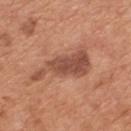Part of a total-body skin-imaging series; this lesion was reviewed on a skin check and was not flagged for biopsy.
Imaged with white-light lighting.
A male subject, aged approximately 65.
Approximately 8 mm at its widest.
A 15 mm crop from a total-body photograph taken for skin-cancer surveillance.
The lesion is located on the mid back.
An algorithmic analysis of the crop reported an area of roughly 15 mm², a shape eccentricity near 0.95, and two-axis asymmetry of about 0.5.